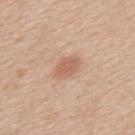  biopsy_status: not biopsied; imaged during a skin examination
  lighting: white-light
  patient:
    sex: male
    age_approx: 60
  automated_metrics:
    area_mm2_approx: 5.0
    shape_asymmetry: 0.15
    cielab_L: 61
    cielab_a: 22
    cielab_b: 31
    vs_skin_darker_L: 9.0
    border_irregularity_0_10: 1.5
    color_variation_0_10: 1.0
    peripheral_color_asymmetry: 0.5
  lesion_size:
    long_diameter_mm_approx: 3.0
  site: upper back
  image:
    source: total-body photography crop
    field_of_view_mm: 15